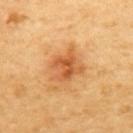biopsy status — no biopsy performed (imaged during a skin exam)